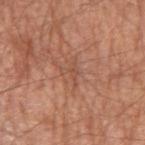This lesion was catalogued during total-body skin photography and was not selected for biopsy. The lesion is on the right upper arm. A 15 mm close-up tile from a total-body photography series done for melanoma screening. A male subject, in their mid- to late 60s.